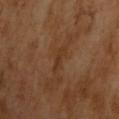A male patient, aged 63–67.
A region of skin cropped from a whole-body photographic capture, roughly 15 mm wide.
This is a cross-polarized tile.
About 3 mm across.